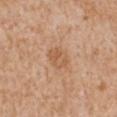| feature | finding |
|---|---|
| lighting | white-light |
| location | the arm |
| diameter | about 3.5 mm |
| subject | male, aged approximately 65 |
| image | 15 mm crop, total-body photography |